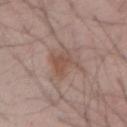Findings:
– notes · total-body-photography surveillance lesion; no biopsy
– location · the left forearm
– image source · ~15 mm tile from a whole-body skin photo
– patient · male, in their mid- to late 40s